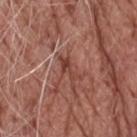biopsy status — imaged on a skin check; not biopsied